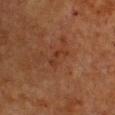| feature | finding |
|---|---|
| workup | no biopsy performed (imaged during a skin exam) |
| illumination | cross-polarized illumination |
| patient | female, aged approximately 55 |
| acquisition | ~15 mm crop, total-body skin-cancer survey |
| anatomic site | the chest |